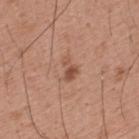Recorded during total-body skin imaging; not selected for excision or biopsy.